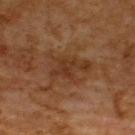Part of a total-body skin-imaging series; this lesion was reviewed on a skin check and was not flagged for biopsy.
A male subject, roughly 60 years of age.
An algorithmic analysis of the crop reported a footprint of about 13 mm² and a shape eccentricity near 0.75. The analysis additionally found a lesion color around L≈33 a*≈19 b*≈29 in CIELAB, about 6 CIELAB-L* units darker than the surrounding skin, and a normalized border contrast of about 6.5. The analysis additionally found a detector confidence of about 100 out of 100 that the crop contains a lesion.
This image is a 15 mm lesion crop taken from a total-body photograph.
On the upper back.
The recorded lesion diameter is about 5.5 mm.